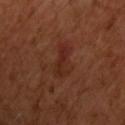Background: The tile uses cross-polarized illumination. On the chest. A close-up tile cropped from a whole-body skin photograph, about 15 mm across. Automated image analysis of the tile measured an average lesion color of about L≈27 a*≈23 b*≈27 (CIELAB) and about 5 CIELAB-L* units darker than the surrounding skin. It also reported a classifier nevus-likeness of about 5/100 and a detector confidence of about 100 out of 100 that the crop contains a lesion. Longest diameter approximately 4 mm. The subject is a male approximately 50 years of age.